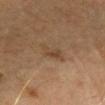* biopsy status · catalogued during a skin exam; not biopsied
* lesion diameter · ≈2.5 mm
* imaging modality · 15 mm crop, total-body photography
* anatomic site · the head or neck
* subject · male, aged 63 to 67
* automated metrics · a lesion area of about 4.5 mm², a shape eccentricity near 0.6, and a symmetry-axis asymmetry near 0.3; an automated nevus-likeness rating near 5 out of 100 and a detector confidence of about 100 out of 100 that the crop contains a lesion